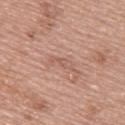Recorded during total-body skin imaging; not selected for excision or biopsy. The patient is a female in their 60s. The lesion is on the upper back. Cropped from a total-body skin-imaging series; the visible field is about 15 mm.